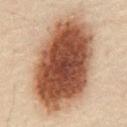Assessment: Imaged during a routine full-body skin examination; the lesion was not biopsied and no histopathology is available. Image and clinical context: A 15 mm crop from a total-body photograph taken for skin-cancer surveillance. The lesion is located on the abdomen. The subject is a male approximately 50 years of age. Automated image analysis of the tile measured a lesion area of about 70 mm². The software also gave radial color variation of about 2.5. It also reported an automated nevus-likeness rating near 100 out of 100 and lesion-presence confidence of about 100/100. Imaged with cross-polarized lighting.